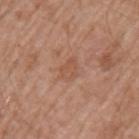Notes:
– biopsy status — imaged on a skin check; not biopsied
– patient — male, roughly 75 years of age
– acquisition — ~15 mm crop, total-body skin-cancer survey
– automated metrics — a within-lesion color-variation index near 1.5/10; lesion-presence confidence of about 100/100
– tile lighting — white-light
– diameter — about 3 mm
– body site — the left upper arm